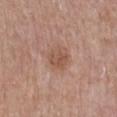Captured during whole-body skin photography for melanoma surveillance; the lesion was not biopsied.
This is a white-light tile.
Measured at roughly 3 mm in maximum diameter.
The lesion is located on the right lower leg.
A female subject in their mid- to late 70s.
A close-up tile cropped from a whole-body skin photograph, about 15 mm across.